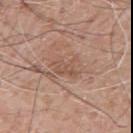biopsy_status: not biopsied; imaged during a skin examination
patient:
  sex: male
  age_approx: 60
site: arm
lesion_size:
  long_diameter_mm_approx: 3.5
image:
  source: total-body photography crop
  field_of_view_mm: 15